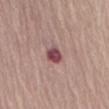{"biopsy_status": "not biopsied; imaged during a skin examination", "site": "abdomen", "lesion_size": {"long_diameter_mm_approx": 2.5}, "automated_metrics": {"area_mm2_approx": 5.0, "eccentricity": 0.55, "shape_asymmetry": 0.2, "border_irregularity_0_10": 1.5, "color_variation_0_10": 4.5, "peripheral_color_asymmetry": 1.5}, "lighting": "white-light", "patient": {"sex": "female", "age_approx": 65}, "image": {"source": "total-body photography crop", "field_of_view_mm": 15}}